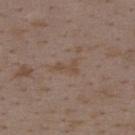biopsy status: total-body-photography surveillance lesion; no biopsy
site: the upper back
lighting: white-light
patient: female, aged 33–37
lesion diameter: ~2.5 mm (longest diameter)
imaging modality: 15 mm crop, total-body photography